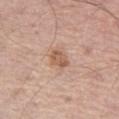Clinical impression: Imaged during a routine full-body skin examination; the lesion was not biopsied and no histopathology is available. Background: Located on the left thigh. The total-body-photography lesion software estimated a lesion color around L≈57 a*≈21 b*≈31 in CIELAB and a normalized lesion–skin contrast near 7.5. The analysis additionally found a border-irregularity index near 2/10, a color-variation rating of about 2/10, and a peripheral color-asymmetry measure near 0.5. A 15 mm crop from a total-body photograph taken for skin-cancer surveillance. The patient is a male approximately 75 years of age. This is a white-light tile. About 2.5 mm across.